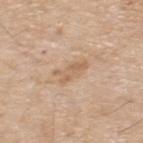Recorded during total-body skin imaging; not selected for excision or biopsy.
A male patient aged around 70.
Located on the upper back.
Cropped from a total-body skin-imaging series; the visible field is about 15 mm.
Automated image analysis of the tile measured a lesion area of about 5.5 mm² and a shape-asymmetry score of about 0.35 (0 = symmetric). The analysis additionally found an average lesion color of about L≈62 a*≈17 b*≈33 (CIELAB), roughly 8 lightness units darker than nearby skin, and a normalized lesion–skin contrast near 5.5. And it measured a border-irregularity index near 4.5/10, internal color variation of about 2 on a 0–10 scale, and a peripheral color-asymmetry measure near 0.5.
The tile uses white-light illumination.